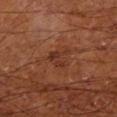Impression: Recorded during total-body skin imaging; not selected for excision or biopsy. Background: This image is a 15 mm lesion crop taken from a total-body photograph. The lesion-visualizer software estimated a lesion area of about 4.5 mm², a shape eccentricity near 0.65, and two-axis asymmetry of about 0.6. The software also gave a mean CIELAB color near L≈32 a*≈23 b*≈29, about 6 CIELAB-L* units darker than the surrounding skin, and a normalized border contrast of about 6. It also reported a classifier nevus-likeness of about 0/100 and a detector confidence of about 100 out of 100 that the crop contains a lesion. From the left lower leg. This is a cross-polarized tile. A male patient in their mid-60s.